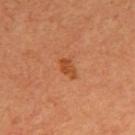biopsy status = catalogued during a skin exam; not biopsied
anatomic site = the upper back
subject = female, aged approximately 65
lesion size = about 2.5 mm
image = ~15 mm tile from a whole-body skin photo